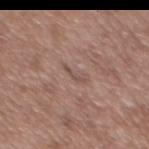Impression:
The lesion was tiled from a total-body skin photograph and was not biopsied.
Image and clinical context:
On the mid back. The lesion's longest dimension is about 2.5 mm. A lesion tile, about 15 mm wide, cut from a 3D total-body photograph. Imaged with white-light lighting. The patient is a male roughly 50 years of age.